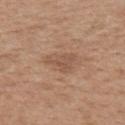<record>
  <site>upper back</site>
  <image>
    <source>total-body photography crop</source>
    <field_of_view_mm>15</field_of_view_mm>
  </image>
  <patient>
    <sex>male</sex>
    <age_approx>30</age_approx>
  </patient>
  <lesion_size>
    <long_diameter_mm_approx>3.5</long_diameter_mm_approx>
  </lesion_size>
</record>